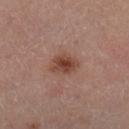The lesion was photographed on a routine skin check and not biopsied; there is no pathology result. About 3.5 mm across. Located on the left lower leg. A male subject, aged 53–57. This image is a 15 mm lesion crop taken from a total-body photograph.